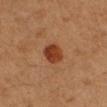This lesion was catalogued during total-body skin photography and was not selected for biopsy.
A 15 mm close-up extracted from a 3D total-body photography capture.
The lesion-visualizer software estimated a mean CIELAB color near L≈33 a*≈23 b*≈31, about 11 CIELAB-L* units darker than the surrounding skin, and a normalized lesion–skin contrast near 10. The software also gave a classifier nevus-likeness of about 100/100 and lesion-presence confidence of about 100/100.
Captured under cross-polarized illumination.
Located on the left forearm.
Longest diameter approximately 3 mm.
The subject is a female in their 40s.